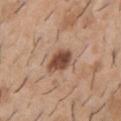workup = no biopsy performed (imaged during a skin exam); body site = the chest; patient = male, aged around 40; image source = 15 mm crop, total-body photography; tile lighting = white-light; size = ~3.5 mm (longest diameter).